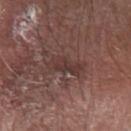{"patient": {"sex": "male", "age_approx": 80}, "image": {"source": "total-body photography crop", "field_of_view_mm": 15}, "site": "left forearm", "automated_metrics": {"area_mm2_approx": 7.5, "shape_asymmetry": 0.25}, "lesion_size": {"long_diameter_mm_approx": 5.0}}